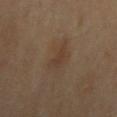Assessment: Imaged during a routine full-body skin examination; the lesion was not biopsied and no histopathology is available. Acquisition and patient details: The lesion is located on the right upper arm. A male subject approximately 85 years of age or older. Approximately 3 mm at its widest. A region of skin cropped from a whole-body photographic capture, roughly 15 mm wide. The tile uses cross-polarized illumination.